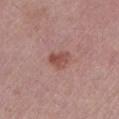Q: Is there a histopathology result?
A: catalogued during a skin exam; not biopsied
Q: What did automated image analysis measure?
A: a lesion area of about 4.5 mm² and an eccentricity of roughly 0.7
Q: What is the imaging modality?
A: total-body-photography crop, ~15 mm field of view
Q: Patient demographics?
A: female, roughly 65 years of age
Q: What is the anatomic site?
A: the leg
Q: How was the tile lit?
A: white-light